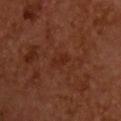{"biopsy_status": "not biopsied; imaged during a skin examination", "site": "upper back", "patient": {"sex": "male", "age_approx": 55}, "lighting": "cross-polarized", "automated_metrics": {"cielab_L": 24, "cielab_a": 23, "cielab_b": 26, "vs_skin_darker_L": 4.0, "vs_skin_contrast_norm": 5.0}, "image": {"source": "total-body photography crop", "field_of_view_mm": 15}, "lesion_size": {"long_diameter_mm_approx": 3.0}}